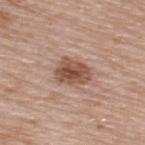The lesion was photographed on a routine skin check and not biopsied; there is no pathology result. The tile uses white-light illumination. Located on the upper back. Longest diameter approximately 4 mm. A region of skin cropped from a whole-body photographic capture, roughly 15 mm wide. A male patient, approximately 65 years of age.